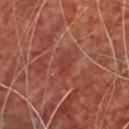biopsy_status: not biopsied; imaged during a skin examination
lighting: cross-polarized
patient:
  sex: male
  age_approx: 65
lesion_size:
  long_diameter_mm_approx: 2.5
image:
  source: total-body photography crop
  field_of_view_mm: 15
site: chest